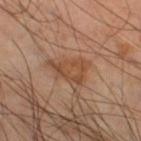<lesion>
<biopsy_status>not biopsied; imaged during a skin examination</biopsy_status>
<automated_metrics>
  <area_mm2_approx>7.5</area_mm2_approx>
  <eccentricity>0.8</eccentricity>
  <border_irregularity_0_10>5.0</border_irregularity_0_10>
  <color_variation_0_10>3.0</color_variation_0_10>
  <peripheral_color_asymmetry>1.0</peripheral_color_asymmetry>
  <nevus_likeness_0_100>5</nevus_likeness_0_100>
  <lesion_detection_confidence_0_100>100</lesion_detection_confidence_0_100>
</automated_metrics>
<image>
  <source>total-body photography crop</source>
  <field_of_view_mm>15</field_of_view_mm>
</image>
<lighting>cross-polarized</lighting>
<lesion_size>
  <long_diameter_mm_approx>4.0</long_diameter_mm_approx>
</lesion_size>
<site>left lower leg</site>
<patient>
  <sex>male</sex>
  <age_approx>50</age_approx>
</patient>
</lesion>